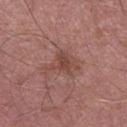– follow-up — imaged on a skin check; not biopsied
– subject — male, approximately 55 years of age
– image — 15 mm crop, total-body photography
– location — the leg